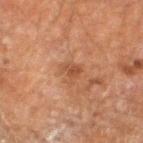lesion size: about 2.5 mm
anatomic site: the left lower leg
lighting: cross-polarized
subject: male, in their 60s
imaging modality: 15 mm crop, total-body photography